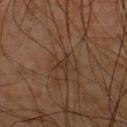Recorded during total-body skin imaging; not selected for excision or biopsy. Cropped from a total-body skin-imaging series; the visible field is about 15 mm. The tile uses cross-polarized illumination. Automated image analysis of the tile measured roughly 6 lightness units darker than nearby skin and a normalized border contrast of about 6. The software also gave a border-irregularity index near 6/10, a color-variation rating of about 1/10, and a peripheral color-asymmetry measure near 0.5. The analysis additionally found an automated nevus-likeness rating near 0 out of 100. A male subject roughly 65 years of age. The lesion is on the chest. Approximately 2.5 mm at its widest.